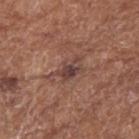The lesion was tiled from a total-body skin photograph and was not biopsied. Imaged with white-light lighting. On the arm. Measured at roughly 4.5 mm in maximum diameter. The patient is a female in their mid- to late 70s. A close-up tile cropped from a whole-body skin photograph, about 15 mm across. The lesion-visualizer software estimated a footprint of about 6.5 mm², an eccentricity of roughly 0.85, and two-axis asymmetry of about 0.45. And it measured an average lesion color of about L≈42 a*≈19 b*≈22 (CIELAB), roughly 9 lightness units darker than nearby skin, and a normalized border contrast of about 7.5. It also reported a border-irregularity rating of about 6/10, a within-lesion color-variation index near 5.5/10, and radial color variation of about 1.5. The software also gave a nevus-likeness score of about 0/100 and a detector confidence of about 95 out of 100 that the crop contains a lesion.